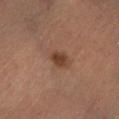The lesion was tiled from a total-body skin photograph and was not biopsied. Located on the left lower leg. The total-body-photography lesion software estimated a lesion area of about 4 mm² and a shape-asymmetry score of about 0.2 (0 = symmetric). And it measured a lesion color around L≈40 a*≈21 b*≈29 in CIELAB. The software also gave a border-irregularity index near 2/10 and internal color variation of about 3 on a 0–10 scale. The lesion's longest dimension is about 2.5 mm. Captured under cross-polarized illumination. A close-up tile cropped from a whole-body skin photograph, about 15 mm across. A male subject approximately 50 years of age.